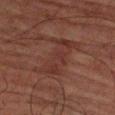Imaged during a routine full-body skin examination; the lesion was not biopsied and no histopathology is available. Longest diameter approximately 6 mm. A male patient, aged 78–82. Captured under cross-polarized illumination. From the right upper arm. This image is a 15 mm lesion crop taken from a total-body photograph.